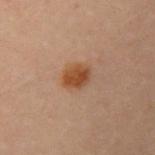Clinical impression:
The lesion was tiled from a total-body skin photograph and was not biopsied.
Background:
A female subject aged around 30. The lesion is located on the left upper arm. This image is a 15 mm lesion crop taken from a total-body photograph. About 3.5 mm across.